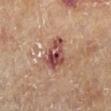Assessment:
The lesion was tiled from a total-body skin photograph and was not biopsied.
Image and clinical context:
Approximately 4 mm at its widest. The subject is a male aged 83 or older. The total-body-photography lesion software estimated a mean CIELAB color near L≈46 a*≈25 b*≈24, roughly 13 lightness units darker than nearby skin, and a normalized border contrast of about 10.5. From the right lower leg. A lesion tile, about 15 mm wide, cut from a 3D total-body photograph. The tile uses cross-polarized illumination.